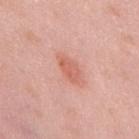Background: The recorded lesion diameter is about 3.5 mm. From the back. A male subject approximately 55 years of age. Cropped from a whole-body photographic skin survey; the tile spans about 15 mm.